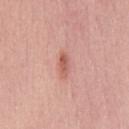- notes · no biopsy performed (imaged during a skin exam)
- diameter · ≈3 mm
- patient · male, aged around 30
- image-analysis metrics · an area of roughly 3 mm² and an eccentricity of roughly 0.9; an average lesion color of about L≈58 a*≈27 b*≈28 (CIELAB); border irregularity of about 2.5 on a 0–10 scale, a color-variation rating of about 1/10, and peripheral color asymmetry of about 0; a classifier nevus-likeness of about 40/100
- tile lighting · white-light illumination
- site · the chest
- imaging modality · total-body-photography crop, ~15 mm field of view